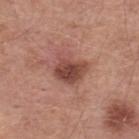follow-up: total-body-photography surveillance lesion; no biopsy | image: ~15 mm tile from a whole-body skin photo | diameter: ~4 mm (longest diameter) | subject: male, about 55 years old | anatomic site: the back | tile lighting: white-light illumination.